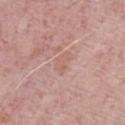<lesion>
  <biopsy_status>not biopsied; imaged during a skin examination</biopsy_status>
  <patient>
    <sex>male</sex>
    <age_approx>55</age_approx>
  </patient>
  <automated_metrics>
    <eccentricity>0.85</eccentricity>
    <shape_asymmetry>0.4</shape_asymmetry>
    <cielab_L>60</cielab_L>
    <cielab_a>21</cielab_a>
    <cielab_b>26</cielab_b>
    <vs_skin_darker_L>6.0</vs_skin_darker_L>
    <vs_skin_contrast_norm>4.5</vs_skin_contrast_norm>
    <color_variation_0_10>1.0</color_variation_0_10>
    <peripheral_color_asymmetry>0.5</peripheral_color_asymmetry>
    <lesion_detection_confidence_0_100>95</lesion_detection_confidence_0_100>
  </automated_metrics>
  <image>
    <source>total-body photography crop</source>
    <field_of_view_mm>15</field_of_view_mm>
  </image>
</lesion>